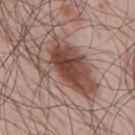workup: total-body-photography surveillance lesion; no biopsy
image-analysis metrics: lesion-presence confidence of about 100/100
lesion diameter: ≈8.5 mm
subject: male, aged 43 to 47
acquisition: 15 mm crop, total-body photography
tile lighting: white-light
location: the back